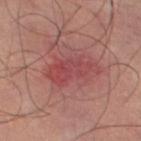This lesion was catalogued during total-body skin photography and was not selected for biopsy.
Located on the left thigh.
Longest diameter approximately 5 mm.
The total-body-photography lesion software estimated an average lesion color of about L≈47 a*≈31 b*≈23 (CIELAB), a lesion–skin lightness drop of about 8, and a normalized lesion–skin contrast near 6. The software also gave a classifier nevus-likeness of about 5/100 and a lesion-detection confidence of about 100/100.
A 15 mm crop from a total-body photograph taken for skin-cancer surveillance.
Imaged with cross-polarized lighting.